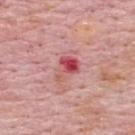biopsy_status: not biopsied; imaged during a skin examination
image:
  source: total-body photography crop
  field_of_view_mm: 15
site: upper back
lighting: white-light
automated_metrics:
  area_mm2_approx: 6.0
  eccentricity: 0.65
  shape_asymmetry: 0.5
  cielab_L: 53
  cielab_a: 36
  cielab_b: 24
  vs_skin_darker_L: 12.0
  vs_skin_contrast_norm: 8.0
patient:
  sex: male
  age_approx: 65
lesion_size:
  long_diameter_mm_approx: 3.5